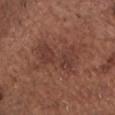Part of a total-body skin-imaging series; this lesion was reviewed on a skin check and was not flagged for biopsy. A 15 mm crop from a total-body photograph taken for skin-cancer surveillance. This is a white-light tile. The lesion is located on the chest. A male patient, aged approximately 75. The lesion-visualizer software estimated a footprint of about 17 mm². And it measured a lesion color around L≈39 a*≈21 b*≈25 in CIELAB and a normalized border contrast of about 6. And it measured a border-irregularity rating of about 5.5/10 and a color-variation rating of about 3/10. Longest diameter approximately 6 mm.